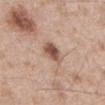Clinical impression: The lesion was photographed on a routine skin check and not biopsied; there is no pathology result. Clinical summary: This is a white-light tile. From the front of the torso. Approximately 3 mm at its widest. Automated tile analysis of the lesion measured a footprint of about 5 mm², a shape eccentricity near 0.7, and a symmetry-axis asymmetry near 0.25. And it measured a color-variation rating of about 4.5/10 and peripheral color asymmetry of about 1.5. The analysis additionally found a nevus-likeness score of about 85/100 and a lesion-detection confidence of about 100/100. A male subject, aged approximately 65. A 15 mm close-up tile from a total-body photography series done for melanoma screening.